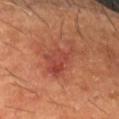Assessment:
Part of a total-body skin-imaging series; this lesion was reviewed on a skin check and was not flagged for biopsy.
Context:
A 15 mm crop from a total-body photograph taken for skin-cancer surveillance. Measured at roughly 6 mm in maximum diameter. The lesion is located on the left forearm. Imaged with cross-polarized lighting. A male patient in their 60s.